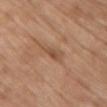<lesion>
<biopsy_status>not biopsied; imaged during a skin examination</biopsy_status>
<site>chest</site>
<automated_metrics>
  <eccentricity>0.9</eccentricity>
  <shape_asymmetry>0.3</shape_asymmetry>
  <cielab_L>51</cielab_L>
  <cielab_a>20</cielab_a>
  <cielab_b>32</cielab_b>
  <vs_skin_darker_L>8.0</vs_skin_darker_L>
  <vs_skin_contrast_norm>6.0</vs_skin_contrast_norm>
  <border_irregularity_0_10>3.5</border_irregularity_0_10>
  <color_variation_0_10>2.5</color_variation_0_10>
</automated_metrics>
<image>
  <source>total-body photography crop</source>
  <field_of_view_mm>15</field_of_view_mm>
</image>
<patient>
  <sex>female</sex>
  <age_approx>65</age_approx>
</patient>
<lighting>white-light</lighting>
</lesion>